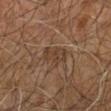Imaged during a routine full-body skin examination; the lesion was not biopsied and no histopathology is available. This image is a 15 mm lesion crop taken from a total-body photograph. A male patient, aged around 60. On the right leg.